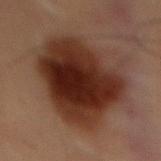Impression: The lesion was tiled from a total-body skin photograph and was not biopsied. Background: Cropped from a total-body skin-imaging series; the visible field is about 15 mm. The lesion is located on the abdomen. An algorithmic analysis of the crop reported a mean CIELAB color near L≈25 a*≈18 b*≈23, about 13 CIELAB-L* units darker than the surrounding skin, and a lesion-to-skin contrast of about 13 (normalized; higher = more distinct). The analysis additionally found border irregularity of about 3 on a 0–10 scale, a within-lesion color-variation index near 7/10, and peripheral color asymmetry of about 2. The analysis additionally found a classifier nevus-likeness of about 100/100 and a lesion-detection confidence of about 100/100. The recorded lesion diameter is about 9.5 mm. The tile uses cross-polarized illumination. A male patient, aged 53 to 57.